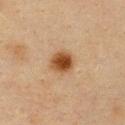The lesion was photographed on a routine skin check and not biopsied; there is no pathology result. A lesion tile, about 15 mm wide, cut from a 3D total-body photograph. A female subject aged 38–42. The lesion is on the chest.